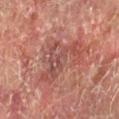No biopsy was performed on this lesion — it was imaged during a full skin examination and was not determined to be concerning. A male subject in their mid-60s. Automated image analysis of the tile measured a footprint of about 17 mm², an eccentricity of roughly 0.75, and two-axis asymmetry of about 0.65. And it measured a mean CIELAB color near L≈49 a*≈26 b*≈25 and a normalized border contrast of about 5.5. And it measured an automated nevus-likeness rating near 0 out of 100 and lesion-presence confidence of about 100/100. Cropped from a whole-body photographic skin survey; the tile spans about 15 mm. Captured under cross-polarized illumination. On the left forearm. Longest diameter approximately 6.5 mm.